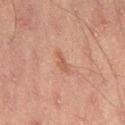biopsy_status: not biopsied; imaged during a skin examination
site: lower back
patient:
  sex: male
  age_approx: 45
image:
  source: total-body photography crop
  field_of_view_mm: 15
lesion_size:
  long_diameter_mm_approx: 2.5
automated_metrics:
  cielab_L: 46
  cielab_a: 21
  cielab_b: 26
  vs_skin_darker_L: 7.0
  vs_skin_contrast_norm: 6.0
  color_variation_0_10: 0.0
  peripheral_color_asymmetry: 0.0